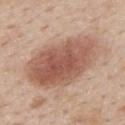An algorithmic analysis of the crop reported a lesion color around L≈56 a*≈21 b*≈28 in CIELAB and a lesion-to-skin contrast of about 8.5 (normalized; higher = more distinct). It also reported a nevus-likeness score of about 100/100 and lesion-presence confidence of about 100/100. A male patient, in their 60s. The tile uses white-light illumination. A lesion tile, about 15 mm wide, cut from a 3D total-body photograph. On the back.